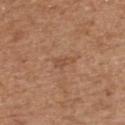biopsy status: imaged on a skin check; not biopsied
imaging modality: total-body-photography crop, ~15 mm field of view
subject: male, roughly 70 years of age
anatomic site: the upper back
illumination: white-light
automated lesion analysis: a lesion area of about 3 mm², an outline eccentricity of about 0.9 (0 = round, 1 = elongated), and a shape-asymmetry score of about 0.35 (0 = symmetric); a mean CIELAB color near L≈50 a*≈22 b*≈32, roughly 7 lightness units darker than nearby skin, and a normalized border contrast of about 5; an automated nevus-likeness rating near 0 out of 100 and a lesion-detection confidence of about 100/100